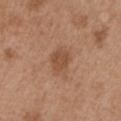Part of a total-body skin-imaging series; this lesion was reviewed on a skin check and was not flagged for biopsy.
Automated tile analysis of the lesion measured a footprint of about 5 mm² and a shape-asymmetry score of about 0.2 (0 = symmetric). The analysis additionally found an automated nevus-likeness rating near 20 out of 100 and a detector confidence of about 100 out of 100 that the crop contains a lesion.
A male patient aged 53–57.
A 15 mm crop from a total-body photograph taken for skin-cancer surveillance.
About 2.5 mm across.
The tile uses white-light illumination.
From the left upper arm.